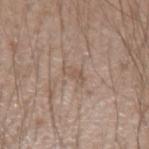workup: imaged on a skin check; not biopsied
tile lighting: white-light
image source: ~15 mm crop, total-body skin-cancer survey
anatomic site: the left forearm
automated lesion analysis: a mean CIELAB color near L≈54 a*≈15 b*≈26, a lesion–skin lightness drop of about 7, and a lesion-to-skin contrast of about 5 (normalized; higher = more distinct); a border-irregularity index near 4.5/10, internal color variation of about 0 on a 0–10 scale, and radial color variation of about 0
patient: male, in their 20s
diameter: ~2.5 mm (longest diameter)